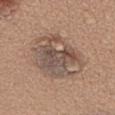follow-up = catalogued during a skin exam; not biopsied | lighting = white-light illumination | image-analysis metrics = a symmetry-axis asymmetry near 0.2; a border-irregularity index near 2/10, internal color variation of about 8.5 on a 0–10 scale, and a peripheral color-asymmetry measure near 3; a classifier nevus-likeness of about 0/100 and a lesion-detection confidence of about 100/100 | acquisition = ~15 mm crop, total-body skin-cancer survey | anatomic site = the upper back | patient = male, in their 60s.